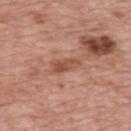Imaged during a routine full-body skin examination; the lesion was not biopsied and no histopathology is available.
A male subject in their 70s.
A close-up tile cropped from a whole-body skin photograph, about 15 mm across.
Located on the back.